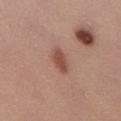  biopsy_status: not biopsied; imaged during a skin examination
  lesion_size:
    long_diameter_mm_approx: 3.5
  lighting: white-light
  image:
    source: total-body photography crop
    field_of_view_mm: 15
  patient:
    sex: male
    age_approx: 30